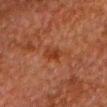Q: Was this lesion biopsied?
A: catalogued during a skin exam; not biopsied
Q: What kind of image is this?
A: 15 mm crop, total-body photography
Q: How large is the lesion?
A: ≈2.5 mm
Q: Lesion location?
A: the chest
Q: What are the patient's age and sex?
A: male, aged 78–82
Q: How was the tile lit?
A: cross-polarized